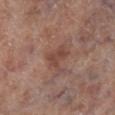Q: Was a biopsy performed?
A: catalogued during a skin exam; not biopsied
Q: What lighting was used for the tile?
A: white-light illumination
Q: What did automated image analysis measure?
A: an average lesion color of about L≈45 a*≈21 b*≈25 (CIELAB) and a lesion–skin lightness drop of about 8; a border-irregularity rating of about 6/10, a within-lesion color-variation index near 2.5/10, and radial color variation of about 1
Q: What are the patient's age and sex?
A: male, about 70 years old
Q: How large is the lesion?
A: ≈3.5 mm
Q: What kind of image is this?
A: 15 mm crop, total-body photography
Q: Where on the body is the lesion?
A: the right lower leg